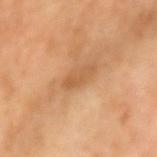size=~3.5 mm (longest diameter) | body site=the right forearm | patient=male, aged around 55 | image=~15 mm crop, total-body skin-cancer survey.